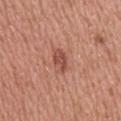biopsy_status: not biopsied; imaged during a skin examination